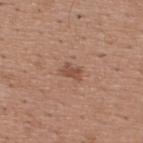Findings:
• follow-up: imaged on a skin check; not biopsied
• tile lighting: white-light
• acquisition: 15 mm crop, total-body photography
• patient: male, in their mid- to late 60s
• anatomic site: the upper back